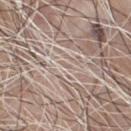<tbp_lesion>
  <biopsy_status>not biopsied; imaged during a skin examination</biopsy_status>
</tbp_lesion>